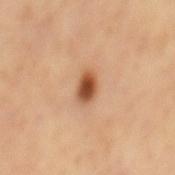– follow-up · imaged on a skin check; not biopsied
– image · ~15 mm crop, total-body skin-cancer survey
– automated lesion analysis · an area of roughly 4.5 mm², an eccentricity of roughly 0.8, and a symmetry-axis asymmetry near 0.3; a mean CIELAB color near L≈49 a*≈23 b*≈35, about 16 CIELAB-L* units darker than the surrounding skin, and a lesion-to-skin contrast of about 11 (normalized; higher = more distinct); a border-irregularity index near 2.5/10, internal color variation of about 5 on a 0–10 scale, and radial color variation of about 1.5; an automated nevus-likeness rating near 100 out of 100
– illumination · cross-polarized illumination
– patient · male, aged approximately 60
– body site · the mid back